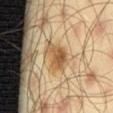follow-up=no biopsy performed (imaged during a skin exam); image source=15 mm crop, total-body photography; anatomic site=the leg; lesion size=about 4 mm; patient=male, aged around 45; lighting=cross-polarized illumination.